Located on the upper back. Measured at roughly 1 mm in maximum diameter. The tile uses white-light illumination. The subject is a male in their mid-50s. Cropped from a whole-body photographic skin survey; the tile spans about 15 mm — 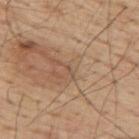Histopathological examination showed a seborrheic keratosis (benign).